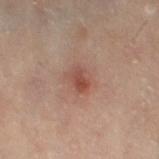Q: Automated lesion metrics?
A: a footprint of about 5.5 mm², a shape eccentricity near 0.55, and two-axis asymmetry of about 0.2; a lesion color around L≈48 a*≈22 b*≈26 in CIELAB and a normalized lesion–skin contrast near 6.5; a within-lesion color-variation index near 4/10; a classifier nevus-likeness of about 35/100
Q: What is the imaging modality?
A: ~15 mm tile from a whole-body skin photo
Q: What are the patient's age and sex?
A: female, about 50 years old
Q: Where on the body is the lesion?
A: the left thigh
Q: How was the tile lit?
A: cross-polarized
Q: How large is the lesion?
A: about 3 mm